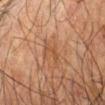Imaged during a routine full-body skin examination; the lesion was not biopsied and no histopathology is available. Imaged with cross-polarized lighting. About 2.5 mm across. A roughly 15 mm field-of-view crop from a total-body skin photograph. The subject is a male aged approximately 80. The lesion is on the right forearm.